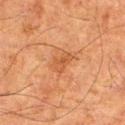biopsy_status: not biopsied; imaged during a skin examination
site: right thigh
automated_metrics:
  area_mm2_approx: 3.5
  eccentricity: 0.8
  shape_asymmetry: 0.3
  cielab_L: 43
  cielab_a: 23
  cielab_b: 34
  vs_skin_darker_L: 7.0
  vs_skin_contrast_norm: 5.5
  nevus_likeness_0_100: 0
  lesion_detection_confidence_0_100: 100
image:
  source: total-body photography crop
  field_of_view_mm: 15
lesion_size:
  long_diameter_mm_approx: 2.5
patient:
  sex: male
  age_approx: 80